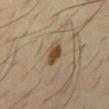Notes:
– lighting · cross-polarized
– acquisition · 15 mm crop, total-body photography
– patient · male, aged 38 to 42
– location · the chest
– automated metrics · roughly 13 lightness units darker than nearby skin and a normalized lesion–skin contrast near 10; a border-irregularity index near 3/10, internal color variation of about 2.5 on a 0–10 scale, and radial color variation of about 0.5; a nevus-likeness score of about 95/100 and a detector confidence of about 100 out of 100 that the crop contains a lesion